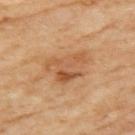Q: Was this lesion biopsied?
A: no biopsy performed (imaged during a skin exam)
Q: Patient demographics?
A: female, in their 60s
Q: Automated lesion metrics?
A: a footprint of about 13 mm², an eccentricity of roughly 0.75, and a symmetry-axis asymmetry near 0.45; an average lesion color of about L≈55 a*≈22 b*≈37 (CIELAB), roughly 9 lightness units darker than nearby skin, and a normalized lesion–skin contrast near 6; a classifier nevus-likeness of about 5/100 and lesion-presence confidence of about 100/100
Q: Lesion location?
A: the left upper arm
Q: What kind of image is this?
A: ~15 mm tile from a whole-body skin photo
Q: Illumination type?
A: cross-polarized illumination
Q: How large is the lesion?
A: ≈5 mm